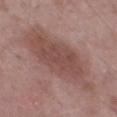Assessment: No biopsy was performed on this lesion — it was imaged during a full skin examination and was not determined to be concerning. Context: The subject is a male roughly 50 years of age. The lesion is on the left thigh. The total-body-photography lesion software estimated a lesion area of about 36 mm², an outline eccentricity of about 0.95 (0 = round, 1 = elongated), and a symmetry-axis asymmetry near 0.25. The software also gave an average lesion color of about L≈49 a*≈20 b*≈22 (CIELAB) and a normalized border contrast of about 7. The lesion's longest dimension is about 11.5 mm. The tile uses white-light illumination. A 15 mm crop from a total-body photograph taken for skin-cancer surveillance.